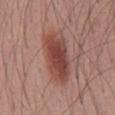Assessment: No biopsy was performed on this lesion — it was imaged during a full skin examination and was not determined to be concerning. Clinical summary: A male patient about 40 years old. The total-body-photography lesion software estimated about 12 CIELAB-L* units darker than the surrounding skin. The analysis additionally found lesion-presence confidence of about 100/100. The lesion is on the chest. A 15 mm close-up extracted from a 3D total-body photography capture. Captured under white-light illumination.